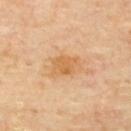The lesion was tiled from a total-body skin photograph and was not biopsied. Automated tile analysis of the lesion measured a mean CIELAB color near L≈64 a*≈21 b*≈43, about 8 CIELAB-L* units darker than the surrounding skin, and a normalized border contrast of about 6.5. The analysis additionally found a border-irregularity index near 2.5/10, internal color variation of about 3 on a 0–10 scale, and peripheral color asymmetry of about 1. About 4 mm across. Captured under cross-polarized illumination. From the upper back. A close-up tile cropped from a whole-body skin photograph, about 15 mm across. A male patient, aged around 65.